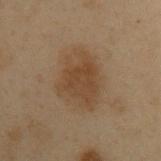This lesion was catalogued during total-body skin photography and was not selected for biopsy. This image is a 15 mm lesion crop taken from a total-body photograph. An algorithmic analysis of the crop reported a footprint of about 23 mm² and an outline eccentricity of about 0.6 (0 = round, 1 = elongated). Located on the upper back. The patient is a male roughly 55 years of age.